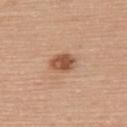follow-up: imaged on a skin check; not biopsied | size: ~3 mm (longest diameter) | imaging modality: 15 mm crop, total-body photography | site: the upper back | illumination: white-light | patient: female, aged around 65.